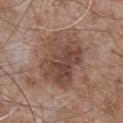Impression:
No biopsy was performed on this lesion — it was imaged during a full skin examination and was not determined to be concerning.
Context:
A 15 mm crop from a total-body photograph taken for skin-cancer surveillance. Captured under white-light illumination. Approximately 6 mm at its widest. From the mid back. The total-body-photography lesion software estimated a lesion area of about 25 mm², an eccentricity of roughly 0.65, and a symmetry-axis asymmetry near 0.2. The analysis additionally found an average lesion color of about L≈45 a*≈18 b*≈25 (CIELAB). It also reported a nevus-likeness score of about 50/100 and lesion-presence confidence of about 100/100. A male patient about 60 years old.